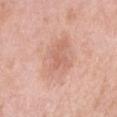Clinical impression:
No biopsy was performed on this lesion — it was imaged during a full skin examination and was not determined to be concerning.
Context:
The lesion is on the arm. The tile uses white-light illumination. Automated tile analysis of the lesion measured an average lesion color of about L≈66 a*≈22 b*≈29 (CIELAB), a lesion–skin lightness drop of about 8, and a lesion-to-skin contrast of about 5 (normalized; higher = more distinct). And it measured a classifier nevus-likeness of about 0/100 and a detector confidence of about 100 out of 100 that the crop contains a lesion. The recorded lesion diameter is about 6.5 mm. A 15 mm crop from a total-body photograph taken for skin-cancer surveillance. A female patient, approximately 30 years of age.